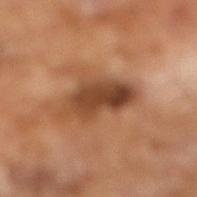Case summary:
* workup: total-body-photography surveillance lesion; no biopsy
* image source: 15 mm crop, total-body photography
* illumination: cross-polarized illumination
* automated metrics: a lesion area of about 14 mm², an outline eccentricity of about 0.85 (0 = round, 1 = elongated), and a symmetry-axis asymmetry near 0.35; an average lesion color of about L≈43 a*≈22 b*≈32 (CIELAB), roughly 13 lightness units darker than nearby skin, and a normalized border contrast of about 10
* patient: male, aged approximately 65